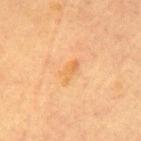Captured during whole-body skin photography for melanoma surveillance; the lesion was not biopsied. The lesion is on the mid back. Longest diameter approximately 3 mm. The subject is a female roughly 60 years of age. A close-up tile cropped from a whole-body skin photograph, about 15 mm across.